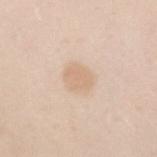biopsy status: no biopsy performed (imaged during a skin exam) | location: the arm | image: total-body-photography crop, ~15 mm field of view | image-analysis metrics: a lesion area of about 5.5 mm² and an outline eccentricity of about 0.6 (0 = round, 1 = elongated); a color-variation rating of about 1.5/10; a classifier nevus-likeness of about 25/100 | patient: female, about 20 years old | diameter: ≈3 mm | lighting: white-light.